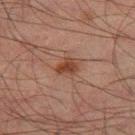Q: Was this lesion biopsied?
A: catalogued during a skin exam; not biopsied
Q: What is the lesion's diameter?
A: ≈2.5 mm
Q: What lighting was used for the tile?
A: cross-polarized
Q: Who is the patient?
A: male, approximately 50 years of age
Q: Where on the body is the lesion?
A: the leg
Q: How was this image acquired?
A: ~15 mm tile from a whole-body skin photo